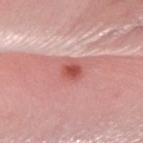biopsy status: total-body-photography surveillance lesion; no biopsy | diameter: about 2.5 mm | automated lesion analysis: a lesion area of about 5 mm², an outline eccentricity of about 0.6 (0 = round, 1 = elongated), and a symmetry-axis asymmetry near 0.2; a nevus-likeness score of about 100/100 and a detector confidence of about 100 out of 100 that the crop contains a lesion | location: the right forearm | lighting: white-light illumination | acquisition: total-body-photography crop, ~15 mm field of view | subject: female, in their mid-20s.